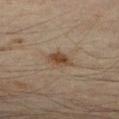notes: total-body-photography surveillance lesion; no biopsy
lighting: cross-polarized illumination
image: 15 mm crop, total-body photography
body site: the right forearm
lesion size: about 2.5 mm
patient: male, approximately 45 years of age